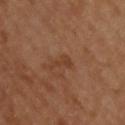Q: Was a biopsy performed?
A: catalogued during a skin exam; not biopsied
Q: How was this image acquired?
A: ~15 mm tile from a whole-body skin photo
Q: Lesion location?
A: the upper back